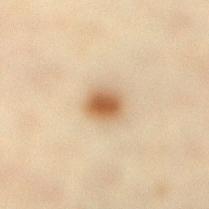A roughly 15 mm field-of-view crop from a total-body skin photograph.
Automated image analysis of the tile measured an eccentricity of roughly 0.5. The software also gave a normalized lesion–skin contrast near 10. And it measured a classifier nevus-likeness of about 100/100 and a lesion-detection confidence of about 100/100.
From the right lower leg.
Longest diameter approximately 3 mm.
A female patient, roughly 65 years of age.